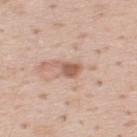Assessment: This lesion was catalogued during total-body skin photography and was not selected for biopsy. Image and clinical context: The recorded lesion diameter is about 3 mm. Located on the back. Cropped from a total-body skin-imaging series; the visible field is about 15 mm. This is a white-light tile. The subject is a male in their mid- to late 30s.